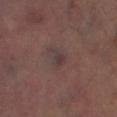Assessment:
Imaged during a routine full-body skin examination; the lesion was not biopsied and no histopathology is available.
Clinical summary:
The tile uses cross-polarized illumination. A 15 mm crop from a total-body photograph taken for skin-cancer surveillance. The lesion-visualizer software estimated a mean CIELAB color near L≈34 a*≈14 b*≈14, roughly 6 lightness units darker than nearby skin, and a normalized lesion–skin contrast near 6.5. On the left lower leg. A male patient, aged 63–67.